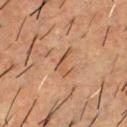Impression:
The lesion was photographed on a routine skin check and not biopsied; there is no pathology result.
Acquisition and patient details:
The lesion-visualizer software estimated a mean CIELAB color near L≈45 a*≈19 b*≈29, roughly 8 lightness units darker than nearby skin, and a normalized border contrast of about 6.5. It also reported a border-irregularity rating of about 8/10, a color-variation rating of about 0/10, and radial color variation of about 0. It also reported a classifier nevus-likeness of about 0/100 and a lesion-detection confidence of about 90/100. A 15 mm close-up tile from a total-body photography series done for melanoma screening. A male subject, approximately 55 years of age. Captured under cross-polarized illumination. Approximately 3.5 mm at its widest. The lesion is located on the upper back.